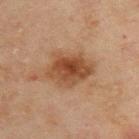Captured during whole-body skin photography for melanoma surveillance; the lesion was not biopsied.
A male subject aged around 45.
An algorithmic analysis of the crop reported a lesion color around L≈41 a*≈19 b*≈30 in CIELAB, roughly 11 lightness units darker than nearby skin, and a normalized border contrast of about 9. And it measured border irregularity of about 2.5 on a 0–10 scale, a within-lesion color-variation index near 5.5/10, and a peripheral color-asymmetry measure near 2.
This is a cross-polarized tile.
The lesion is on the upper back.
The recorded lesion diameter is about 5.5 mm.
A lesion tile, about 15 mm wide, cut from a 3D total-body photograph.